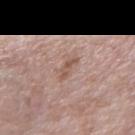anatomic site: the left upper arm | subject: male, aged approximately 60 | image: total-body-photography crop, ~15 mm field of view | automated metrics: a footprint of about 3 mm², an eccentricity of roughly 0.9, and a shape-asymmetry score of about 0.4 (0 = symmetric); a lesion color around L≈54 a*≈19 b*≈26 in CIELAB, roughly 8 lightness units darker than nearby skin, and a lesion-to-skin contrast of about 6.5 (normalized; higher = more distinct); border irregularity of about 4 on a 0–10 scale, a color-variation rating of about 0/10, and peripheral color asymmetry of about 0; an automated nevus-likeness rating near 0 out of 100 and lesion-presence confidence of about 100/100 | tile lighting: white-light | diameter: about 3 mm.